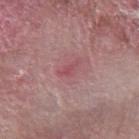biopsy status = total-body-photography surveillance lesion; no biopsy | anatomic site = the left forearm | lighting = white-light illumination | image source = 15 mm crop, total-body photography | patient = male, aged approximately 60 | size = ~3 mm (longest diameter) | image-analysis metrics = a lesion area of about 3 mm² and an eccentricity of roughly 0.9; a mean CIELAB color near L≈51 a*≈28 b*≈18, roughly 7 lightness units darker than nearby skin, and a normalized lesion–skin contrast near 5; a border-irregularity rating of about 5/10 and a within-lesion color-variation index near 0.5/10.